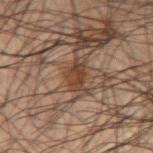Q: What is the imaging modality?
A: ~15 mm tile from a whole-body skin photo
Q: What lighting was used for the tile?
A: cross-polarized illumination
Q: Where on the body is the lesion?
A: the arm
Q: Who is the patient?
A: male, in their mid- to late 40s